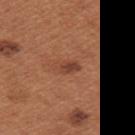notes = no biopsy performed (imaged during a skin exam) | image = 15 mm crop, total-body photography | tile lighting = white-light | subject = female, aged 28 to 32 | site = the upper back | size = ≈3 mm.